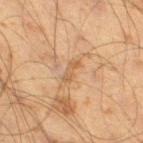Notes:
* workup: catalogued during a skin exam; not biopsied
* TBP lesion metrics: a footprint of about 3 mm², an outline eccentricity of about 0.9 (0 = round, 1 = elongated), and two-axis asymmetry of about 0.35; roughly 6 lightness units darker than nearby skin and a lesion-to-skin contrast of about 5 (normalized; higher = more distinct); a border-irregularity index near 4/10, internal color variation of about 0 on a 0–10 scale, and radial color variation of about 0; an automated nevus-likeness rating near 0 out of 100 and lesion-presence confidence of about 90/100
* imaging modality: ~15 mm crop, total-body skin-cancer survey
* tile lighting: cross-polarized illumination
* body site: the right thigh
* patient: male, approximately 60 years of age
* diameter: ≈3 mm